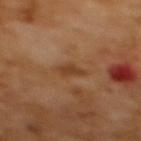Captured during whole-body skin photography for melanoma surveillance; the lesion was not biopsied. A lesion tile, about 15 mm wide, cut from a 3D total-body photograph. The total-body-photography lesion software estimated an area of roughly 4 mm² and a shape-asymmetry score of about 0.3 (0 = symmetric). It also reported a lesion color around L≈42 a*≈21 b*≈35 in CIELAB, roughly 8 lightness units darker than nearby skin, and a normalized lesion–skin contrast near 6.5. The lesion is on the upper back. About 3 mm across. The patient is a female aged approximately 55. Imaged with cross-polarized lighting.